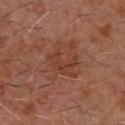The lesion was photographed on a routine skin check and not biopsied; there is no pathology result.
A male subject about 60 years old.
The tile uses cross-polarized illumination.
The lesion is on the chest.
A close-up tile cropped from a whole-body skin photograph, about 15 mm across.